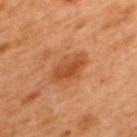notes: imaged on a skin check; not biopsied
tile lighting: cross-polarized
patient: male, in their 50s
location: the upper back
acquisition: total-body-photography crop, ~15 mm field of view
lesion size: about 5 mm